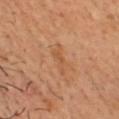biopsy status — no biopsy performed (imaged during a skin exam)
location — the chest
automated metrics — an average lesion color of about L≈54 a*≈23 b*≈37 (CIELAB), about 6 CIELAB-L* units darker than the surrounding skin, and a lesion-to-skin contrast of about 5 (normalized; higher = more distinct); a classifier nevus-likeness of about 0/100
tile lighting — cross-polarized
subject — male, in their 40s
image — 15 mm crop, total-body photography